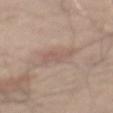Part of a total-body skin-imaging series; this lesion was reviewed on a skin check and was not flagged for biopsy.
From the mid back.
A 15 mm crop from a total-body photograph taken for skin-cancer surveillance.
A male patient, in their mid- to late 60s.
Captured under white-light illumination.